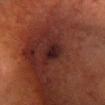Recorded during total-body skin imaging; not selected for excision or biopsy.
The lesion is located on the head or neck.
This is a cross-polarized tile.
Longest diameter approximately 3.5 mm.
This image is a 15 mm lesion crop taken from a total-body photograph.
An algorithmic analysis of the crop reported a lesion color around L≈16 a*≈18 b*≈15 in CIELAB.
The patient is a female roughly 80 years of age.